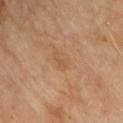biopsy status — no biopsy performed (imaged during a skin exam) | acquisition — ~15 mm crop, total-body skin-cancer survey | diameter — ≈2.5 mm | anatomic site — the front of the torso | tile lighting — cross-polarized illumination | patient — male, aged around 70.